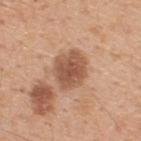Case summary:
– biopsy status — catalogued during a skin exam; not biopsied
– image source — 15 mm crop, total-body photography
– tile lighting — white-light illumination
– size — ≈4 mm
– body site — the upper back
– TBP lesion metrics — a lesion area of about 12 mm²; an average lesion color of about L≈54 a*≈22 b*≈32 (CIELAB), roughly 13 lightness units darker than nearby skin, and a normalized lesion–skin contrast near 8.5; a border-irregularity rating of about 1.5/10, a within-lesion color-variation index near 3/10, and peripheral color asymmetry of about 1; an automated nevus-likeness rating near 10 out of 100
– subject — male, aged around 50